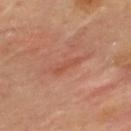workup: total-body-photography surveillance lesion; no biopsy
body site: the back
image: ~15 mm crop, total-body skin-cancer survey
lesion size: ~4 mm (longest diameter)
automated lesion analysis: a footprint of about 4 mm², a shape eccentricity near 0.95, and two-axis asymmetry of about 0.35; border irregularity of about 4.5 on a 0–10 scale, internal color variation of about 0.5 on a 0–10 scale, and a peripheral color-asymmetry measure near 0
subject: female, in their mid-30s